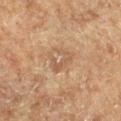No biopsy was performed on this lesion — it was imaged during a full skin examination and was not determined to be concerning.
From the right lower leg.
Longest diameter approximately 3.5 mm.
A male subject, in their mid-70s.
A roughly 15 mm field-of-view crop from a total-body skin photograph.